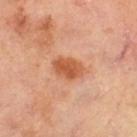notes = no biopsy performed (imaged during a skin exam)
imaging modality = total-body-photography crop, ~15 mm field of view
lesion size = ≈4 mm
subject = male, about 65 years old
automated metrics = a border-irregularity index near 1.5/10, internal color variation of about 3.5 on a 0–10 scale, and radial color variation of about 1; an automated nevus-likeness rating near 90 out of 100 and lesion-presence confidence of about 100/100